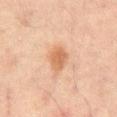follow-up — total-body-photography surveillance lesion; no biopsy
acquisition — ~15 mm tile from a whole-body skin photo
tile lighting — cross-polarized illumination
patient — male, aged 63 to 67
body site — the back
lesion diameter — ~3 mm (longest diameter)